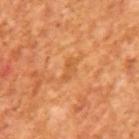Recorded during total-body skin imaging; not selected for excision or biopsy.
The lesion-visualizer software estimated an area of roughly 3 mm², a shape eccentricity near 0.9, and a shape-asymmetry score of about 0.35 (0 = symmetric). The analysis additionally found a peripheral color-asymmetry measure near 0.
Longest diameter approximately 3 mm.
A close-up tile cropped from a whole-body skin photograph, about 15 mm across.
The tile uses cross-polarized illumination.
A male patient aged around 65.